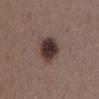Impression: No biopsy was performed on this lesion — it was imaged during a full skin examination and was not determined to be concerning. Image and clinical context: Cropped from a whole-body photographic skin survey; the tile spans about 15 mm. From the mid back. Longest diameter approximately 5 mm. A female subject, aged 33 to 37. Automated tile analysis of the lesion measured an area of roughly 10 mm² and a symmetry-axis asymmetry near 0.1. The software also gave a mean CIELAB color near L≈35 a*≈15 b*≈19, roughly 15 lightness units darker than nearby skin, and a normalized border contrast of about 13. It also reported border irregularity of about 1.5 on a 0–10 scale and a peripheral color-asymmetry measure near 1.5. The tile uses white-light illumination.